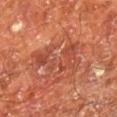Measured at roughly 6.5 mm in maximum diameter. A male patient in their mid-60s. Located on the right lower leg. The total-body-photography lesion software estimated a mean CIELAB color near L≈46 a*≈30 b*≈32 and a normalized border contrast of about 5.5. And it measured internal color variation of about 4.5 on a 0–10 scale and radial color variation of about 1.5. It also reported a nevus-likeness score of about 0/100 and a detector confidence of about 100 out of 100 that the crop contains a lesion. A lesion tile, about 15 mm wide, cut from a 3D total-body photograph.